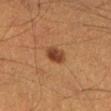Q: Was a biopsy performed?
A: imaged on a skin check; not biopsied
Q: Patient demographics?
A: male, in their 40s
Q: Illumination type?
A: cross-polarized illumination
Q: How large is the lesion?
A: ≈3 mm
Q: Where on the body is the lesion?
A: the left lower leg
Q: What did automated image analysis measure?
A: an average lesion color of about L≈34 a*≈20 b*≈29 (CIELAB) and about 10 CIELAB-L* units darker than the surrounding skin; a peripheral color-asymmetry measure near 1
Q: What kind of image is this?
A: total-body-photography crop, ~15 mm field of view